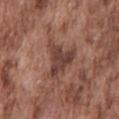Q: Is there a histopathology result?
A: catalogued during a skin exam; not biopsied
Q: What is the anatomic site?
A: the mid back
Q: What is the imaging modality?
A: 15 mm crop, total-body photography
Q: Who is the patient?
A: male, in their mid-70s
Q: What lighting was used for the tile?
A: white-light illumination